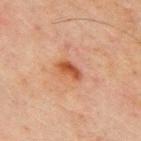workup — imaged on a skin check; not biopsied.